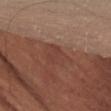<lesion>
  <biopsy_status>not biopsied; imaged during a skin examination</biopsy_status>
  <lighting>cross-polarized</lighting>
  <image>
    <source>total-body photography crop</source>
    <field_of_view_mm>15</field_of_view_mm>
  </image>
  <site>left thigh</site>
  <patient>
    <sex>female</sex>
    <age_approx>45</age_approx>
  </patient>
</lesion>